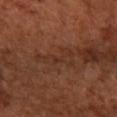<record>
<biopsy_status>not biopsied; imaged during a skin examination</biopsy_status>
<site>right forearm</site>
<lighting>cross-polarized</lighting>
<lesion_size>
  <long_diameter_mm_approx>4.5</long_diameter_mm_approx>
</lesion_size>
<patient>
  <sex>female</sex>
  <age_approx>65</age_approx>
</patient>
<image>
  <source>total-body photography crop</source>
  <field_of_view_mm>15</field_of_view_mm>
</image>
</record>